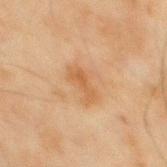<lesion>
<image>
  <source>total-body photography crop</source>
  <field_of_view_mm>15</field_of_view_mm>
</image>
<site>back</site>
<automated_metrics>
  <cielab_L>49</cielab_L>
  <cielab_a>17</cielab_a>
  <cielab_b>33</cielab_b>
  <vs_skin_contrast_norm>5.5</vs_skin_contrast_norm>
  <nevus_likeness_0_100>0</nevus_likeness_0_100>
</automated_metrics>
<lighting>cross-polarized</lighting>
<patient>
  <sex>male</sex>
  <age_approx>45</age_approx>
</patient>
</lesion>